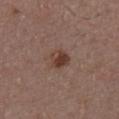No biopsy was performed on this lesion — it was imaged during a full skin examination and was not determined to be concerning. Cropped from a total-body skin-imaging series; the visible field is about 15 mm. The lesion-visualizer software estimated a footprint of about 5 mm² and a shape-asymmetry score of about 0.2 (0 = symmetric). And it measured a mean CIELAB color near L≈39 a*≈19 b*≈24, a lesion–skin lightness drop of about 10, and a lesion-to-skin contrast of about 8.5 (normalized; higher = more distinct). The software also gave a nevus-likeness score of about 90/100. The subject is a male aged 38 to 42. On the chest. Imaged with white-light lighting.